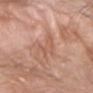Q: What are the patient's age and sex?
A: male, aged around 60
Q: How large is the lesion?
A: ~3.5 mm (longest diameter)
Q: What is the anatomic site?
A: the left forearm
Q: What is the imaging modality?
A: ~15 mm tile from a whole-body skin photo
Q: How was the tile lit?
A: white-light